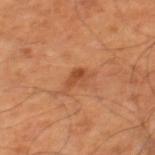No biopsy was performed on this lesion — it was imaged during a full skin examination and was not determined to be concerning.
Located on the right thigh.
Longest diameter approximately 2.5 mm.
Automated image analysis of the tile measured an area of roughly 3 mm², an outline eccentricity of about 0.8 (0 = round, 1 = elongated), and a shape-asymmetry score of about 0.35 (0 = symmetric). The analysis additionally found an average lesion color of about L≈48 a*≈27 b*≈38 (CIELAB), about 9 CIELAB-L* units darker than the surrounding skin, and a normalized border contrast of about 7.
A patient aged around 65.
The tile uses cross-polarized illumination.
A 15 mm close-up extracted from a 3D total-body photography capture.